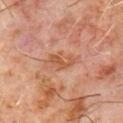Clinical impression:
This lesion was catalogued during total-body skin photography and was not selected for biopsy.
Context:
Cropped from a total-body skin-imaging series; the visible field is about 15 mm. The lesion is on the chest. Approximately 3.5 mm at its widest. This is a cross-polarized tile. The subject is a male in their 60s.